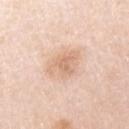Captured during whole-body skin photography for melanoma surveillance; the lesion was not biopsied.
This is a white-light tile.
The subject is a female aged around 45.
Automated image analysis of the tile measured an average lesion color of about L≈71 a*≈19 b*≈32 (CIELAB), roughly 9 lightness units darker than nearby skin, and a normalized lesion–skin contrast near 6. The software also gave a nevus-likeness score of about 25/100 and lesion-presence confidence of about 100/100.
The lesion is on the right upper arm.
A roughly 15 mm field-of-view crop from a total-body skin photograph.